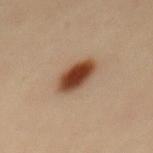Part of a total-body skin-imaging series; this lesion was reviewed on a skin check and was not flagged for biopsy. Automated image analysis of the tile measured a nevus-likeness score of about 100/100. About 4.5 mm across. A female patient, aged around 35. The lesion is located on the mid back. This image is a 15 mm lesion crop taken from a total-body photograph. This is a cross-polarized tile.